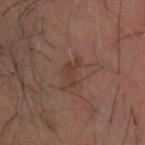Clinical impression: Imaged during a routine full-body skin examination; the lesion was not biopsied and no histopathology is available. Context: The lesion is located on the head or neck. A 15 mm close-up extracted from a 3D total-body photography capture. A male subject about 65 years old. Imaged with cross-polarized lighting. Automated image analysis of the tile measured a shape eccentricity near 0.85 and a symmetry-axis asymmetry near 0.6. The analysis additionally found a lesion color around L≈38 a*≈18 b*≈25 in CIELAB, a lesion–skin lightness drop of about 6, and a normalized lesion–skin contrast near 5.5. The software also gave border irregularity of about 7.5 on a 0–10 scale, internal color variation of about 1 on a 0–10 scale, and peripheral color asymmetry of about 0. The analysis additionally found a classifier nevus-likeness of about 0/100 and a lesion-detection confidence of about 100/100. The recorded lesion diameter is about 3 mm.